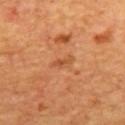Impression: The lesion was tiled from a total-body skin photograph and was not biopsied. Background: A male subject, aged approximately 65. Located on the back. Cropped from a whole-body photographic skin survey; the tile spans about 15 mm.